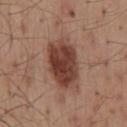Case summary:
- biopsy status: no biopsy performed (imaged during a skin exam)
- automated metrics: an area of roughly 19 mm² and an eccentricity of roughly 0.8
- lesion diameter: about 6.5 mm
- acquisition: ~15 mm crop, total-body skin-cancer survey
- illumination: white-light
- subject: male, aged 53 to 57
- location: the back